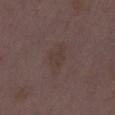follow-up — total-body-photography surveillance lesion; no biopsy | body site — the left thigh | image source — 15 mm crop, total-body photography | subject — female, in their mid-30s.